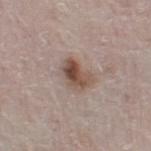Part of a total-body skin-imaging series; this lesion was reviewed on a skin check and was not flagged for biopsy. A region of skin cropped from a whole-body photographic capture, roughly 15 mm wide. On the left lower leg. Automated image analysis of the tile measured an area of roughly 7.5 mm² and two-axis asymmetry of about 0.3. The analysis additionally found an average lesion color of about L≈50 a*≈16 b*≈25 (CIELAB), a lesion–skin lightness drop of about 12, and a normalized lesion–skin contrast near 9.5. The analysis additionally found a border-irregularity index near 3/10. A male subject aged 78 to 82. Longest diameter approximately 3.5 mm.